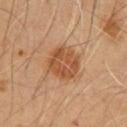The lesion was photographed on a routine skin check and not biopsied; there is no pathology result.
The subject is a male aged 53–57.
Imaged with cross-polarized lighting.
Cropped from a total-body skin-imaging series; the visible field is about 15 mm.
An algorithmic analysis of the crop reported an area of roughly 13 mm², an outline eccentricity of about 0.45 (0 = round, 1 = elongated), and a shape-asymmetry score of about 0.15 (0 = symmetric). The software also gave an average lesion color of about L≈50 a*≈22 b*≈35 (CIELAB), a lesion–skin lightness drop of about 10, and a lesion-to-skin contrast of about 7.5 (normalized; higher = more distinct). It also reported a border-irregularity index near 1.5/10, a color-variation rating of about 4/10, and peripheral color asymmetry of about 1.5.
From the chest.
The lesion's longest dimension is about 4.5 mm.